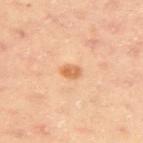No biopsy was performed on this lesion — it was imaged during a full skin examination and was not determined to be concerning.
A close-up tile cropped from a whole-body skin photograph, about 15 mm across.
A female subject aged 38–42.
On the arm.